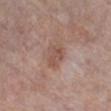Q: Was this lesion biopsied?
A: catalogued during a skin exam; not biopsied
Q: Lesion size?
A: ~3.5 mm (longest diameter)
Q: Lesion location?
A: the right lower leg
Q: What kind of image is this?
A: 15 mm crop, total-body photography
Q: Patient demographics?
A: female, about 70 years old
Q: Illumination type?
A: white-light illumination